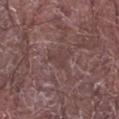Impression:
Imaged during a routine full-body skin examination; the lesion was not biopsied and no histopathology is available.
Image and clinical context:
This is a white-light tile. The patient is a male aged 63–67. A 15 mm close-up extracted from a 3D total-body photography capture. On the right forearm. Measured at roughly 2.5 mm in maximum diameter. The total-body-photography lesion software estimated a lesion color around L≈40 a*≈19 b*≈18 in CIELAB, a lesion–skin lightness drop of about 6, and a normalized border contrast of about 5. The software also gave lesion-presence confidence of about 60/100.